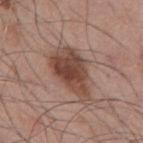workup = total-body-photography surveillance lesion; no biopsy | tile lighting = white-light | site = the back | image source = 15 mm crop, total-body photography | size = ≈6.5 mm | patient = male, approximately 55 years of age.